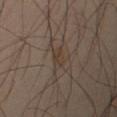subject — male, about 40 years old
imaging modality — 15 mm crop, total-body photography
tile lighting — cross-polarized illumination
diameter — ≈2.5 mm
body site — the right upper arm
image-analysis metrics — an area of roughly 4 mm² and a symmetry-axis asymmetry near 0.25; a peripheral color-asymmetry measure near 0.5; a classifier nevus-likeness of about 0/100 and lesion-presence confidence of about 80/100